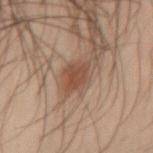Q: Was a biopsy performed?
A: total-body-photography surveillance lesion; no biopsy
Q: What is the imaging modality?
A: 15 mm crop, total-body photography
Q: Lesion size?
A: ~3 mm (longest diameter)
Q: What is the anatomic site?
A: the left arm
Q: Who is the patient?
A: male, aged 48–52
Q: How was the tile lit?
A: cross-polarized illumination